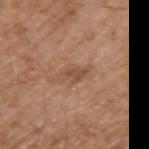Assessment:
The lesion was photographed on a routine skin check and not biopsied; there is no pathology result.
Acquisition and patient details:
Automated tile analysis of the lesion measured a footprint of about 4.5 mm². The analysis additionally found a mean CIELAB color near L≈50 a*≈19 b*≈31 and roughly 8 lightness units darker than nearby skin. The analysis additionally found an automated nevus-likeness rating near 0 out of 100. A male patient, in their mid- to late 70s. A roughly 15 mm field-of-view crop from a total-body skin photograph. The lesion is located on the left upper arm. The lesion's longest dimension is about 3.5 mm.